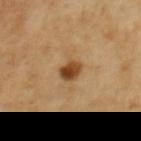Case summary:
– workup: imaged on a skin check; not biopsied
– site: the chest
– size: ~2.5 mm (longest diameter)
– patient: female, in their mid- to late 50s
– illumination: cross-polarized illumination
– acquisition: ~15 mm crop, total-body skin-cancer survey